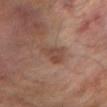notes: no biopsy performed (imaged during a skin exam)
patient: male, about 65 years old
lighting: cross-polarized illumination
acquisition: ~15 mm tile from a whole-body skin photo
site: the right forearm
diameter: about 4 mm